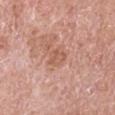Clinical impression: Imaged during a routine full-body skin examination; the lesion was not biopsied and no histopathology is available. Context: A male patient roughly 65 years of age. On the right upper arm. A 15 mm close-up extracted from a 3D total-body photography capture. The lesion's longest dimension is about 2.5 mm. An algorithmic analysis of the crop reported an outline eccentricity of about 0.65 (0 = round, 1 = elongated) and two-axis asymmetry of about 0.4.